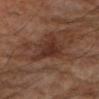Assessment:
Captured during whole-body skin photography for melanoma surveillance; the lesion was not biopsied.
Background:
This is a cross-polarized tile. The lesion's longest dimension is about 4.5 mm. Automated tile analysis of the lesion measured a lesion area of about 9.5 mm². And it measured a border-irregularity rating of about 5/10, a color-variation rating of about 3/10, and radial color variation of about 1. A male patient approximately 85 years of age or older. A lesion tile, about 15 mm wide, cut from a 3D total-body photograph. From the arm.